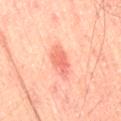{
  "biopsy_status": "not biopsied; imaged during a skin examination",
  "image": {
    "source": "total-body photography crop",
    "field_of_view_mm": 15
  },
  "site": "leg",
  "patient": {
    "sex": "male",
    "age_approx": 65
  },
  "lighting": "cross-polarized",
  "lesion_size": {
    "long_diameter_mm_approx": 4.0
  }
}